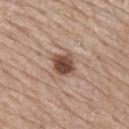Assessment: This lesion was catalogued during total-body skin photography and was not selected for biopsy. Image and clinical context: The lesion is located on the mid back. The tile uses white-light illumination. The recorded lesion diameter is about 3 mm. A male subject, roughly 80 years of age. A 15 mm crop from a total-body photograph taken for skin-cancer surveillance.